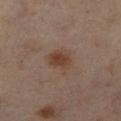Background:
From the left lower leg. A 15 mm close-up extracted from a 3D total-body photography capture. The tile uses cross-polarized illumination. An algorithmic analysis of the crop reported about 7 CIELAB-L* units darker than the surrounding skin and a normalized border contrast of about 7.5. Longest diameter approximately 3.5 mm. A female patient about 50 years old.